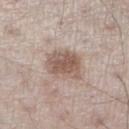This lesion was catalogued during total-body skin photography and was not selected for biopsy.
A 15 mm close-up tile from a total-body photography series done for melanoma screening.
The lesion is located on the leg.
Measured at roughly 4 mm in maximum diameter.
The total-body-photography lesion software estimated an average lesion color of about L≈56 a*≈16 b*≈24 (CIELAB), roughly 12 lightness units darker than nearby skin, and a normalized lesion–skin contrast near 8. And it measured a border-irregularity rating of about 2/10, a color-variation rating of about 4/10, and peripheral color asymmetry of about 1.5.
A male subject, aged 58 to 62.
The tile uses white-light illumination.